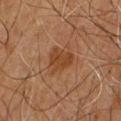follow-up: no biopsy performed (imaged during a skin exam) | site: the chest | TBP lesion metrics: a footprint of about 8 mm², an eccentricity of roughly 0.55, and a symmetry-axis asymmetry near 0.35; a border-irregularity index near 3/10, a color-variation rating of about 2/10, and peripheral color asymmetry of about 0.5; an automated nevus-likeness rating near 5 out of 100 and a lesion-detection confidence of about 100/100 | illumination: cross-polarized | imaging modality: 15 mm crop, total-body photography | lesion size: ≈3.5 mm | patient: male, aged around 65.